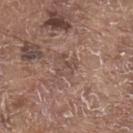Impression:
Captured during whole-body skin photography for melanoma surveillance; the lesion was not biopsied.
Context:
An algorithmic analysis of the crop reported a shape eccentricity near 0.4 and a symmetry-axis asymmetry near 0.7. And it measured border irregularity of about 8 on a 0–10 scale, a within-lesion color-variation index near 3/10, and radial color variation of about 1.5. Captured under white-light illumination. On the upper back. The subject is a male in their 80s. Measured at roughly 2.5 mm in maximum diameter. A lesion tile, about 15 mm wide, cut from a 3D total-body photograph.